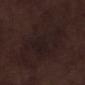Q: Was this lesion biopsied?
A: no biopsy performed (imaged during a skin exam)
Q: Who is the patient?
A: male, aged around 70
Q: What lighting was used for the tile?
A: white-light
Q: What is the lesion's diameter?
A: ≈10.5 mm
Q: What is the imaging modality?
A: 15 mm crop, total-body photography
Q: Where on the body is the lesion?
A: the left lower leg